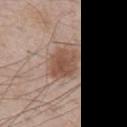workup = catalogued during a skin exam; not biopsied
lesion size = ≈4 mm
subject = male, aged around 55
lighting = white-light
acquisition = ~15 mm tile from a whole-body skin photo